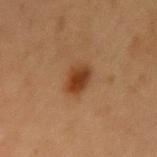Notes:
• biopsy status — no biopsy performed (imaged during a skin exam)
• tile lighting — cross-polarized illumination
• TBP lesion metrics — a lesion color around L≈35 a*≈20 b*≈31 in CIELAB, a lesion–skin lightness drop of about 10, and a lesion-to-skin contrast of about 9.5 (normalized; higher = more distinct); a lesion-detection confidence of about 100/100
• diameter — ~3.5 mm (longest diameter)
• anatomic site — the left upper arm
• acquisition — total-body-photography crop, ~15 mm field of view
• patient — female, in their 40s